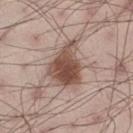| key | value |
|---|---|
| follow-up | imaged on a skin check; not biopsied |
| patient | male, about 30 years old |
| imaging modality | ~15 mm crop, total-body skin-cancer survey |
| image-analysis metrics | a footprint of about 17 mm², an outline eccentricity of about 0.65 (0 = round, 1 = elongated), and two-axis asymmetry of about 0.35; a lesion color around L≈51 a*≈18 b*≈25 in CIELAB, roughly 14 lightness units darker than nearby skin, and a lesion-to-skin contrast of about 10 (normalized; higher = more distinct); a detector confidence of about 100 out of 100 that the crop contains a lesion |
| body site | the leg |
| size | about 6 mm |